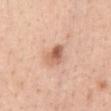Captured during whole-body skin photography for melanoma surveillance; the lesion was not biopsied.
A female subject, approximately 55 years of age.
A lesion tile, about 15 mm wide, cut from a 3D total-body photograph.
About 3 mm across.
Located on the front of the torso.